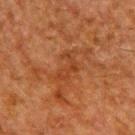Approximately 3.5 mm at its widest.
The lesion-visualizer software estimated a border-irregularity index near 7.5/10, internal color variation of about 1 on a 0–10 scale, and a peripheral color-asymmetry measure near 0. And it measured a nevus-likeness score of about 0/100.
A male subject aged 58–62.
A 15 mm close-up tile from a total-body photography series done for melanoma screening.
The lesion is located on the upper back.
Imaged with cross-polarized lighting.